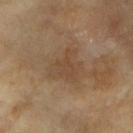biopsy status = imaged on a skin check; not biopsied | illumination = cross-polarized | acquisition = total-body-photography crop, ~15 mm field of view | patient = female, approximately 75 years of age | anatomic site = the right forearm.